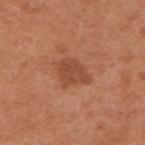| key | value |
|---|---|
| biopsy status | total-body-photography surveillance lesion; no biopsy |
| body site | the upper back |
| patient | male, aged 53–57 |
| acquisition | total-body-photography crop, ~15 mm field of view |
| TBP lesion metrics | a footprint of about 8 mm² and two-axis asymmetry of about 0.3; a nevus-likeness score of about 15/100 and lesion-presence confidence of about 100/100 |
| tile lighting | white-light illumination |